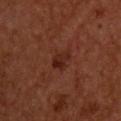  biopsy_status: not biopsied; imaged during a skin examination
  patient:
    sex: male
    age_approx: 55
  site: upper back
  image:
    source: total-body photography crop
    field_of_view_mm: 15